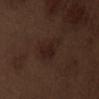Notes:
– image source — ~15 mm tile from a whole-body skin photo
– lesion size — ~3.5 mm (longest diameter)
– image-analysis metrics — a color-variation rating of about 2.5/10 and peripheral color asymmetry of about 0.5
– location — the abdomen
– patient — male, about 70 years old
– tile lighting — white-light